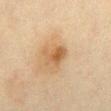Case summary:
• biopsy status: imaged on a skin check; not biopsied